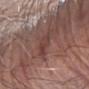Q: Is there a histopathology result?
A: catalogued during a skin exam; not biopsied
Q: What is the anatomic site?
A: the right forearm
Q: Lesion size?
A: ~3 mm (longest diameter)
Q: How was the tile lit?
A: white-light
Q: Patient demographics?
A: male, aged 73 to 77
Q: How was this image acquired?
A: 15 mm crop, total-body photography
Q: Automated lesion metrics?
A: border irregularity of about 3 on a 0–10 scale and internal color variation of about 3 on a 0–10 scale